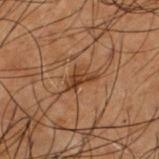notes: catalogued during a skin exam; not biopsied
acquisition: ~15 mm crop, total-body skin-cancer survey
patient: male, aged approximately 50
diameter: about 3 mm
tile lighting: cross-polarized illumination
location: the upper back